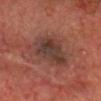notes: no biopsy performed (imaged during a skin exam)
diameter: about 7 mm
subject: male, approximately 75 years of age
location: the chest
imaging modality: ~15 mm crop, total-body skin-cancer survey
illumination: cross-polarized